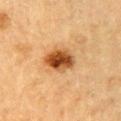Findings:
• biopsy status: total-body-photography surveillance lesion; no biopsy
• image: ~15 mm crop, total-body skin-cancer survey
• lesion size: about 4 mm
• subject: male, aged 83 or older
• anatomic site: the arm
• TBP lesion metrics: two-axis asymmetry of about 0.2; an average lesion color of about L≈45 a*≈23 b*≈38 (CIELAB), about 16 CIELAB-L* units darker than the surrounding skin, and a normalized border contrast of about 11.5; a border-irregularity index near 1.5/10, a within-lesion color-variation index near 7.5/10, and a peripheral color-asymmetry measure near 2; an automated nevus-likeness rating near 100 out of 100